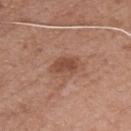Recorded during total-body skin imaging; not selected for excision or biopsy. Longest diameter approximately 3.5 mm. A male subject about 75 years old. Imaged with white-light lighting. A 15 mm crop from a total-body photograph taken for skin-cancer surveillance. An algorithmic analysis of the crop reported border irregularity of about 3 on a 0–10 scale, a within-lesion color-variation index near 2/10, and radial color variation of about 0.5. Located on the front of the torso.